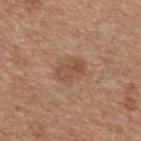Recorded during total-body skin imaging; not selected for excision or biopsy. The lesion is located on the left upper arm. This is a white-light tile. About 3.5 mm across. A female patient aged 48–52. A 15 mm close-up extracted from a 3D total-body photography capture.